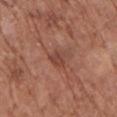The lesion was tiled from a total-body skin photograph and was not biopsied.
The patient is a female roughly 75 years of age.
A 15 mm close-up tile from a total-body photography series done for melanoma screening.
On the upper back.